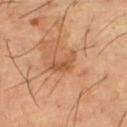| feature | finding |
|---|---|
| biopsy status | no biopsy performed (imaged during a skin exam) |
| lesion size | ≈4 mm |
| subject | male, roughly 65 years of age |
| body site | the left thigh |
| image source | total-body-photography crop, ~15 mm field of view |